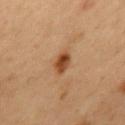• workup · no biopsy performed (imaged during a skin exam)
• patient · male, aged 53 to 57
• lesion diameter · about 2.5 mm
• imaging modality · 15 mm crop, total-body photography
• body site · the mid back
• TBP lesion metrics · a lesion color around L≈37 a*≈20 b*≈31 in CIELAB, a lesion–skin lightness drop of about 11, and a normalized lesion–skin contrast near 10; an automated nevus-likeness rating near 95 out of 100 and a lesion-detection confidence of about 100/100
• illumination · cross-polarized